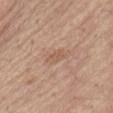Clinical impression:
The lesion was photographed on a routine skin check and not biopsied; there is no pathology result.
Image and clinical context:
The tile uses white-light illumination. About 3 mm across. A roughly 15 mm field-of-view crop from a total-body skin photograph. The subject is a male aged around 70. On the abdomen.